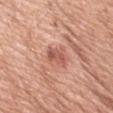follow-up: total-body-photography surveillance lesion; no biopsy.